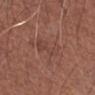biopsy_status: not biopsied; imaged during a skin examination
patient:
  sex: male
  age_approx: 65
site: left forearm
lesion_size:
  long_diameter_mm_approx: 4.0
image:
  source: total-body photography crop
  field_of_view_mm: 15
lighting: white-light